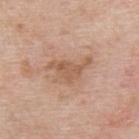<lesion>
  <biopsy_status>not biopsied; imaged during a skin examination</biopsy_status>
  <patient>
    <sex>male</sex>
    <age_approx>60</age_approx>
  </patient>
  <image>
    <source>total-body photography crop</source>
    <field_of_view_mm>15</field_of_view_mm>
  </image>
  <site>upper back</site>
</lesion>